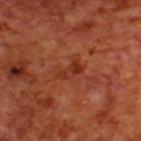Recorded during total-body skin imaging; not selected for excision or biopsy. The patient is a male in their 70s. From the upper back. The lesion's longest dimension is about 3 mm. Captured under cross-polarized illumination. A lesion tile, about 15 mm wide, cut from a 3D total-body photograph.